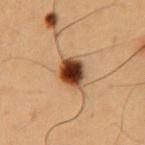Cropped from a whole-body photographic skin survey; the tile spans about 15 mm.
A male subject, aged 48 to 52.
The tile uses cross-polarized illumination.
On the chest.